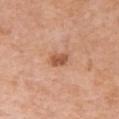No biopsy was performed on this lesion — it was imaged during a full skin examination and was not determined to be concerning.
Imaged with white-light lighting.
Longest diameter approximately 2.5 mm.
A female subject, aged 53–57.
The lesion is located on the right upper arm.
A roughly 15 mm field-of-view crop from a total-body skin photograph.